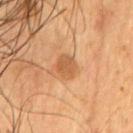notes=imaged on a skin check; not biopsied
lesion size=≈2.5 mm
anatomic site=the chest
lighting=cross-polarized illumination
patient=male, roughly 55 years of age
TBP lesion metrics=a lesion area of about 5.5 mm² and two-axis asymmetry of about 0.2; a classifier nevus-likeness of about 20/100
acquisition=total-body-photography crop, ~15 mm field of view